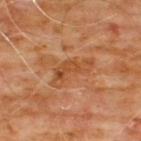Impression: Imaged during a routine full-body skin examination; the lesion was not biopsied and no histopathology is available. Background: A lesion tile, about 15 mm wide, cut from a 3D total-body photograph. Located on the chest. A male patient, approximately 60 years of age.